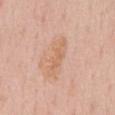The lesion was photographed on a routine skin check and not biopsied; there is no pathology result. The lesion's longest dimension is about 5.5 mm. The lesion is on the mid back. This is a white-light tile. A male subject, in their mid-50s. Cropped from a total-body skin-imaging series; the visible field is about 15 mm.